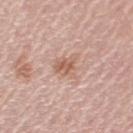Notes:
• workup: catalogued during a skin exam; not biopsied
• image: 15 mm crop, total-body photography
• body site: the left upper arm
• lesion diameter: about 3.5 mm
• patient: female, about 65 years old